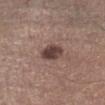<lesion>
  <biopsy_status>not biopsied; imaged during a skin examination</biopsy_status>
  <lesion_size>
    <long_diameter_mm_approx>2.5</long_diameter_mm_approx>
  </lesion_size>
  <image>
    <source>total-body photography crop</source>
    <field_of_view_mm>15</field_of_view_mm>
  </image>
  <site>right lower leg</site>
  <lighting>white-light</lighting>
  <patient>
    <sex>male</sex>
    <age_approx>55</age_approx>
  </patient>
</lesion>